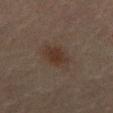workup = imaged on a skin check; not biopsied | subject = male, roughly 70 years of age | lesion diameter = about 4 mm | automated lesion analysis = an eccentricity of roughly 0.6; a border-irregularity rating of about 2/10, a within-lesion color-variation index near 2.5/10, and peripheral color asymmetry of about 1; a classifier nevus-likeness of about 80/100 and a detector confidence of about 100 out of 100 that the crop contains a lesion | anatomic site = the abdomen | image source = total-body-photography crop, ~15 mm field of view.